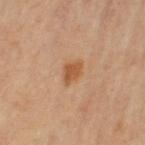Case summary:
- follow-up — catalogued during a skin exam; not biopsied
- image source — 15 mm crop, total-body photography
- TBP lesion metrics — a lesion area of about 4 mm², an outline eccentricity of about 0.75 (0 = round, 1 = elongated), and a shape-asymmetry score of about 0.35 (0 = symmetric); a border-irregularity rating of about 3/10, internal color variation of about 1.5 on a 0–10 scale, and peripheral color asymmetry of about 0.5
- lighting — cross-polarized illumination
- diameter — ~2.5 mm (longest diameter)
- anatomic site — the left upper arm
- patient — female, aged 63 to 67